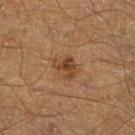{"biopsy_status": "not biopsied; imaged during a skin examination", "lesion_size": {"long_diameter_mm_approx": 2.5}, "lighting": "cross-polarized", "image": {"source": "total-body photography crop", "field_of_view_mm": 15}, "patient": {"sex": "male", "age_approx": 65}, "automated_metrics": {"eccentricity": 0.55, "shape_asymmetry": 0.35}, "site": "leg"}